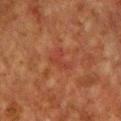A male subject, approximately 60 years of age. Cropped from a whole-body photographic skin survey; the tile spans about 15 mm. On the chest. Imaged with cross-polarized lighting.